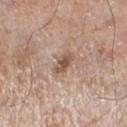workup: no biopsy performed (imaged during a skin exam) | subject: male, roughly 80 years of age | acquisition: ~15 mm crop, total-body skin-cancer survey | image-analysis metrics: a footprint of about 4 mm² and two-axis asymmetry of about 0.25; a within-lesion color-variation index near 2.5/10; an automated nevus-likeness rating near 55 out of 100 | lighting: white-light illumination | anatomic site: the left lower leg | diameter: ~3 mm (longest diameter).